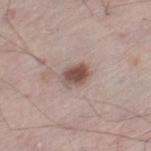lighting: white-light
image:
  source: total-body photography crop
  field_of_view_mm: 15
site: left thigh
patient:
  sex: male
  age_approx: 55
automated_metrics:
  vs_skin_darker_L: 14.0
  vs_skin_contrast_norm: 9.5
  border_irregularity_0_10: 1.5
  color_variation_0_10: 4.5
  peripheral_color_asymmetry: 1.5
  nevus_likeness_0_100: 95
lesion_size:
  long_diameter_mm_approx: 3.0